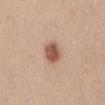The lesion was photographed on a routine skin check and not biopsied; there is no pathology result.
An algorithmic analysis of the crop reported a lesion area of about 6 mm². The analysis additionally found a mean CIELAB color near L≈55 a*≈21 b*≈29. And it measured border irregularity of about 1 on a 0–10 scale. The analysis additionally found a classifier nevus-likeness of about 100/100 and a lesion-detection confidence of about 100/100.
The lesion is located on the front of the torso.
Measured at roughly 3 mm in maximum diameter.
A 15 mm close-up extracted from a 3D total-body photography capture.
The patient is a female about 40 years old.
The tile uses white-light illumination.